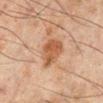| key | value |
|---|---|
| workup | no biopsy performed (imaged during a skin exam) |
| TBP lesion metrics | a border-irregularity rating of about 2.5/10, a within-lesion color-variation index near 3/10, and radial color variation of about 1; a nevus-likeness score of about 75/100 |
| lesion diameter | ≈4 mm |
| site | the right lower leg |
| subject | male, aged 43–47 |
| acquisition | total-body-photography crop, ~15 mm field of view |